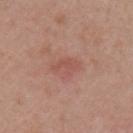follow-up — imaged on a skin check; not biopsied
site — the left upper arm
acquisition — 15 mm crop, total-body photography
patient — female, approximately 45 years of age